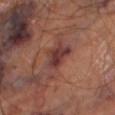Q: Is there a histopathology result?
A: total-body-photography surveillance lesion; no biopsy
Q: What kind of image is this?
A: 15 mm crop, total-body photography
Q: Lesion location?
A: the right thigh
Q: How large is the lesion?
A: about 5.5 mm
Q: Patient demographics?
A: male, aged around 65
Q: What lighting was used for the tile?
A: cross-polarized illumination